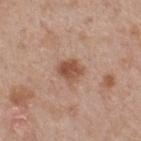diameter=~3 mm (longest diameter)
acquisition=~15 mm tile from a whole-body skin photo
automated lesion analysis=a border-irregularity index near 2/10, a within-lesion color-variation index near 4/10, and peripheral color asymmetry of about 1.5; a lesion-detection confidence of about 100/100
illumination=white-light illumination
subject=male, approximately 55 years of age